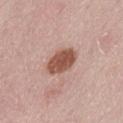<lesion>
<biopsy_status>not biopsied; imaged during a skin examination</biopsy_status>
<site>abdomen</site>
<image>
  <source>total-body photography crop</source>
  <field_of_view_mm>15</field_of_view_mm>
</image>
<patient>
  <sex>female</sex>
  <age_approx>30</age_approx>
</patient>
</lesion>